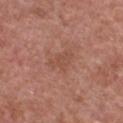Case summary:
* follow-up: total-body-photography surveillance lesion; no biopsy
* imaging modality: total-body-photography crop, ~15 mm field of view
* size: ≈3.5 mm
* subject: male, aged around 65
* automated metrics: a lesion area of about 5.5 mm², a shape eccentricity near 0.75, and two-axis asymmetry of about 0.4
* anatomic site: the chest